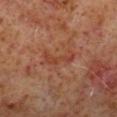patient = male, aged 58–62 | TBP lesion metrics = a footprint of about 5.5 mm², an eccentricity of roughly 0.95, and a symmetry-axis asymmetry near 0.45 | body site = the right lower leg | imaging modality = ~15 mm tile from a whole-body skin photo | illumination = cross-polarized illumination.